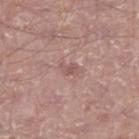This lesion was catalogued during total-body skin photography and was not selected for biopsy.
On the right lower leg.
Captured under white-light illumination.
About 2.5 mm across.
A male patient, about 65 years old.
A lesion tile, about 15 mm wide, cut from a 3D total-body photograph.